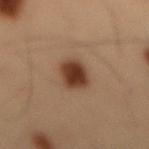No biopsy was performed on this lesion — it was imaged during a full skin examination and was not determined to be concerning.
Located on the lower back.
A male subject in their mid-50s.
The lesion-visualizer software estimated a lesion color around L≈30 a*≈17 b*≈24 in CIELAB, about 13 CIELAB-L* units darker than the surrounding skin, and a lesion-to-skin contrast of about 12 (normalized; higher = more distinct). And it measured a border-irregularity index near 1.5/10, a color-variation rating of about 3/10, and radial color variation of about 0.5. The software also gave a nevus-likeness score of about 100/100.
This image is a 15 mm lesion crop taken from a total-body photograph.
This is a cross-polarized tile.